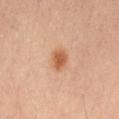Assessment: Captured during whole-body skin photography for melanoma surveillance; the lesion was not biopsied. Clinical summary: A lesion tile, about 15 mm wide, cut from a 3D total-body photograph. From the leg. The subject is a male in their mid-50s.